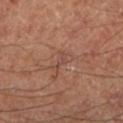Q: Was a biopsy performed?
A: imaged on a skin check; not biopsied
Q: Illumination type?
A: cross-polarized
Q: Where on the body is the lesion?
A: the left lower leg
Q: Automated lesion metrics?
A: a shape eccentricity near 0.9
Q: How was this image acquired?
A: ~15 mm crop, total-body skin-cancer survey
Q: Patient demographics?
A: male, aged 63 to 67
Q: How large is the lesion?
A: about 3 mm